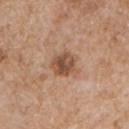Case summary:
* workup · catalogued during a skin exam; not biopsied
* site · the left upper arm
* subject · male, approximately 65 years of age
* image source · ~15 mm tile from a whole-body skin photo